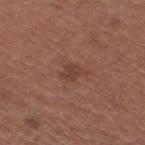Impression: The lesion was photographed on a routine skin check and not biopsied; there is no pathology result. Context: The lesion is located on the right thigh. Automated tile analysis of the lesion measured a footprint of about 3.5 mm², an outline eccentricity of about 0.65 (0 = round, 1 = elongated), and a symmetry-axis asymmetry near 0.4. The analysis additionally found a lesion color around L≈41 a*≈20 b*≈25 in CIELAB, a lesion–skin lightness drop of about 7, and a normalized lesion–skin contrast near 6. Imaged with white-light lighting. The lesion's longest dimension is about 2.5 mm. A close-up tile cropped from a whole-body skin photograph, about 15 mm across. The patient is a female aged 53 to 57.